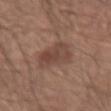No biopsy was performed on this lesion — it was imaged during a full skin examination and was not determined to be concerning.
An algorithmic analysis of the crop reported an outline eccentricity of about 0.85 (0 = round, 1 = elongated) and a shape-asymmetry score of about 0.3 (0 = symmetric). The analysis additionally found a border-irregularity index near 3.5/10, a color-variation rating of about 4/10, and peripheral color asymmetry of about 1.5.
The tile uses white-light illumination.
A roughly 15 mm field-of-view crop from a total-body skin photograph.
The lesion is on the right forearm.
The recorded lesion diameter is about 5.5 mm.
A male patient in their 40s.